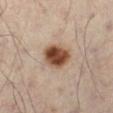{"biopsy_status": "not biopsied; imaged during a skin examination", "image": {"source": "total-body photography crop", "field_of_view_mm": 15}, "lesion_size": {"long_diameter_mm_approx": 4.0}, "patient": {"sex": "male", "age_approx": 50}, "site": "left leg", "lighting": "cross-polarized"}